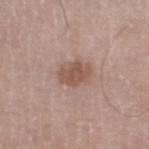Clinical impression:
Part of a total-body skin-imaging series; this lesion was reviewed on a skin check and was not flagged for biopsy.
Image and clinical context:
An algorithmic analysis of the crop reported a lesion area of about 7 mm², an eccentricity of roughly 0.7, and a symmetry-axis asymmetry near 0.25. The software also gave a lesion–skin lightness drop of about 10 and a normalized lesion–skin contrast near 7.5. The lesion is on the leg. A 15 mm close-up extracted from a 3D total-body photography capture. Captured under white-light illumination. A male subject in their mid-60s.